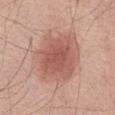Imaged during a routine full-body skin examination; the lesion was not biopsied and no histopathology is available. A male patient roughly 70 years of age. The lesion is located on the abdomen. Cropped from a whole-body photographic skin survey; the tile spans about 15 mm.